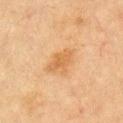Context: Located on the left upper arm. Imaged with cross-polarized lighting. A male patient, aged 63–67. A region of skin cropped from a whole-body photographic capture, roughly 15 mm wide.